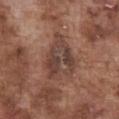Q: Was this lesion biopsied?
A: total-body-photography surveillance lesion; no biopsy
Q: Patient demographics?
A: male, in their mid-70s
Q: What did automated image analysis measure?
A: a footprint of about 17 mm² and an outline eccentricity of about 0.7 (0 = round, 1 = elongated); border irregularity of about 4 on a 0–10 scale, a color-variation rating of about 6.5/10, and radial color variation of about 2; a classifier nevus-likeness of about 0/100 and a lesion-detection confidence of about 75/100
Q: What is the lesion's diameter?
A: ~6 mm (longest diameter)
Q: Lesion location?
A: the chest
Q: What is the imaging modality?
A: total-body-photography crop, ~15 mm field of view